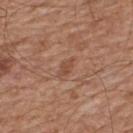Imaged during a routine full-body skin examination; the lesion was not biopsied and no histopathology is available.
Located on the upper back.
This is a white-light tile.
The recorded lesion diameter is about 2.5 mm.
The total-body-photography lesion software estimated a footprint of about 2.5 mm², an outline eccentricity of about 0.85 (0 = round, 1 = elongated), and a symmetry-axis asymmetry near 0.3. It also reported a lesion-to-skin contrast of about 6 (normalized; higher = more distinct). The software also gave border irregularity of about 3 on a 0–10 scale and a color-variation rating of about 0.5/10. And it measured an automated nevus-likeness rating near 0 out of 100 and a lesion-detection confidence of about 100/100.
A male patient aged approximately 65.
A 15 mm crop from a total-body photograph taken for skin-cancer surveillance.